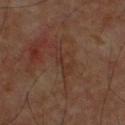Assessment:
The lesion was photographed on a routine skin check and not biopsied; there is no pathology result.
Image and clinical context:
Automated tile analysis of the lesion measured a footprint of about 3 mm², an outline eccentricity of about 0.95 (0 = round, 1 = elongated), and a shape-asymmetry score of about 0.4 (0 = symmetric). The software also gave a detector confidence of about 90 out of 100 that the crop contains a lesion. On the chest. A region of skin cropped from a whole-body photographic capture, roughly 15 mm wide. The patient is a male aged 58 to 62. Imaged with cross-polarized lighting. About 3.5 mm across.